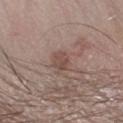| feature | finding |
|---|---|
| follow-up | imaged on a skin check; not biopsied |
| site | the right forearm |
| illumination | white-light illumination |
| TBP lesion metrics | border irregularity of about 2.5 on a 0–10 scale and internal color variation of about 2 on a 0–10 scale; an automated nevus-likeness rating near 5 out of 100 and a lesion-detection confidence of about 100/100 |
| image source | ~15 mm tile from a whole-body skin photo |
| subject | male, approximately 75 years of age |